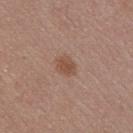Case summary:
- size — ≈2.5 mm
- patient — male, about 25 years old
- acquisition — total-body-photography crop, ~15 mm field of view
- location — the chest
- illumination — white-light